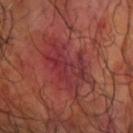{"biopsy_status": "not biopsied; imaged during a skin examination", "lesion_size": {"long_diameter_mm_approx": 6.0}, "patient": {"sex": "male", "age_approx": 60}, "lighting": "cross-polarized", "automated_metrics": {"area_mm2_approx": 16.0, "shape_asymmetry": 0.4, "border_irregularity_0_10": 6.5, "color_variation_0_10": 6.0, "peripheral_color_asymmetry": 2.5, "lesion_detection_confidence_0_100": 85}, "site": "right forearm", "image": {"source": "total-body photography crop", "field_of_view_mm": 15}}